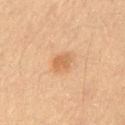The lesion was photographed on a routine skin check and not biopsied; there is no pathology result.